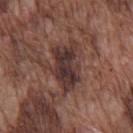<tbp_lesion>
<biopsy_status>not biopsied; imaged during a skin examination</biopsy_status>
<patient>
  <sex>male</sex>
  <age_approx>75</age_approx>
</patient>
<site>front of the torso</site>
<image>
  <source>total-body photography crop</source>
  <field_of_view_mm>15</field_of_view_mm>
</image>
</tbp_lesion>